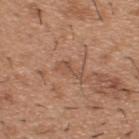Clinical impression:
This lesion was catalogued during total-body skin photography and was not selected for biopsy.
Acquisition and patient details:
Approximately 3 mm at its widest. Located on the upper back. This image is a 15 mm lesion crop taken from a total-body photograph. This is a white-light tile. A male patient approximately 40 years of age. An algorithmic analysis of the crop reported a lesion area of about 3.5 mm², an outline eccentricity of about 0.9 (0 = round, 1 = elongated), and a symmetry-axis asymmetry near 0.45. The analysis additionally found a classifier nevus-likeness of about 0/100 and lesion-presence confidence of about 90/100.